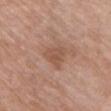<case>
<biopsy_status>not biopsied; imaged during a skin examination</biopsy_status>
<image>
  <source>total-body photography crop</source>
  <field_of_view_mm>15</field_of_view_mm>
</image>
<site>chest</site>
<automated_metrics>
  <area_mm2_approx>6.0</area_mm2_approx>
  <eccentricity>0.55</eccentricity>
  <shape_asymmetry>0.4</shape_asymmetry>
  <border_irregularity_0_10>3.5</border_irregularity_0_10>
  <color_variation_0_10>2.5</color_variation_0_10>
  <peripheral_color_asymmetry>1.0</peripheral_color_asymmetry>
</automated_metrics>
<patient>
  <sex>female</sex>
  <age_approx>60</age_approx>
</patient>
<lesion_size>
  <long_diameter_mm_approx>3.0</long_diameter_mm_approx>
</lesion_size>
</case>